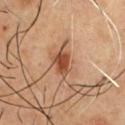Assessment:
The lesion was photographed on a routine skin check and not biopsied; there is no pathology result.
Image and clinical context:
A male patient aged 53 to 57. Cropped from a whole-body photographic skin survey; the tile spans about 15 mm. The total-body-photography lesion software estimated an area of roughly 5.5 mm² and a shape-asymmetry score of about 0.2 (0 = symmetric). The analysis additionally found a border-irregularity rating of about 2.5/10. The lesion is located on the chest. Approximately 3.5 mm at its widest.